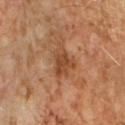follow-up: total-body-photography surveillance lesion; no biopsy | acquisition: total-body-photography crop, ~15 mm field of view | anatomic site: the arm | TBP lesion metrics: a mean CIELAB color near L≈43 a*≈22 b*≈33, roughly 9 lightness units darker than nearby skin, and a normalized lesion–skin contrast near 7; border irregularity of about 4 on a 0–10 scale, internal color variation of about 2.5 on a 0–10 scale, and a peripheral color-asymmetry measure near 1; a classifier nevus-likeness of about 0/100 | subject: male, in their 60s.